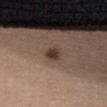<lesion>
  <biopsy_status>not biopsied; imaged during a skin examination</biopsy_status>
  <site>chest</site>
  <lesion_size>
    <long_diameter_mm_approx>2.5</long_diameter_mm_approx>
  </lesion_size>
  <patient>
    <sex>female</sex>
    <age_approx>35</age_approx>
  </patient>
  <lighting>white-light</lighting>
  <image>
    <source>total-body photography crop</source>
    <field_of_view_mm>15</field_of_view_mm>
  </image>
</lesion>